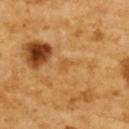* follow-up: total-body-photography surveillance lesion; no biopsy
* acquisition: total-body-photography crop, ~15 mm field of view
* body site: the upper back
* patient: female, aged 53–57